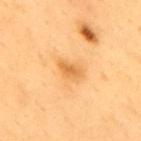follow-up: total-body-photography surveillance lesion; no biopsy | location: the upper back | image source: total-body-photography crop, ~15 mm field of view | diameter: about 3 mm | patient: male, aged 58–62 | automated lesion analysis: a footprint of about 4 mm², a shape eccentricity near 0.85, and a shape-asymmetry score of about 0.35 (0 = symmetric); a border-irregularity rating of about 3/10 and peripheral color asymmetry of about 0.5; a detector confidence of about 100 out of 100 that the crop contains a lesion | illumination: cross-polarized.